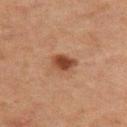workup: catalogued during a skin exam; not biopsied
subject: male, in their 60s
anatomic site: the upper back
image source: ~15 mm tile from a whole-body skin photo
lesion diameter: about 3.5 mm